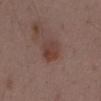This lesion was catalogued during total-body skin photography and was not selected for biopsy. Captured under white-light illumination. A male patient in their 50s. A 15 mm close-up extracted from a 3D total-body photography capture. On the mid back.